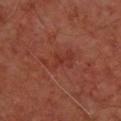{
  "biopsy_status": "not biopsied; imaged during a skin examination"
}